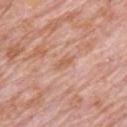The lesion was tiled from a total-body skin photograph and was not biopsied.
The lesion's longest dimension is about 2.5 mm.
A 15 mm close-up tile from a total-body photography series done for melanoma screening.
On the chest.
A male subject, aged around 80.
This is a white-light tile.